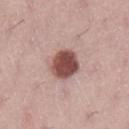Q: Was this lesion biopsied?
A: total-body-photography surveillance lesion; no biopsy
Q: Illumination type?
A: white-light illumination
Q: What did automated image analysis measure?
A: a footprint of about 10 mm², a shape eccentricity near 0.55, and a shape-asymmetry score of about 0.15 (0 = symmetric); a mean CIELAB color near L≈49 a*≈23 b*≈23 and a normalized lesion–skin contrast near 12; border irregularity of about 1.5 on a 0–10 scale, a color-variation rating of about 4/10, and radial color variation of about 1; an automated nevus-likeness rating near 90 out of 100
Q: Where on the body is the lesion?
A: the left lower leg
Q: What is the imaging modality?
A: ~15 mm crop, total-body skin-cancer survey
Q: What are the patient's age and sex?
A: female, aged around 50